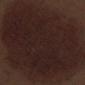Cropped from a whole-body photographic skin survey; the tile spans about 15 mm. Located on the left thigh. Approximately 20 mm at its widest. A male subject aged approximately 70. The total-body-photography lesion software estimated a mean CIELAB color near L≈21 a*≈15 b*≈16, a lesion–skin lightness drop of about 7, and a normalized lesion–skin contrast near 10. It also reported border irregularity of about 2 on a 0–10 scale and peripheral color asymmetry of about 1. And it measured an automated nevus-likeness rating near 0 out of 100.